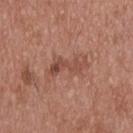Findings:
– workup · no biopsy performed (imaged during a skin exam)
– imaging modality · ~15 mm crop, total-body skin-cancer survey
– lesion diameter · ≈4.5 mm
– anatomic site · the upper back
– subject · male, aged 53 to 57